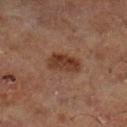The lesion was tiled from a total-body skin photograph and was not biopsied.
A male subject, aged 63–67.
A 15 mm close-up tile from a total-body photography series done for melanoma screening.
Longest diameter approximately 3.5 mm.
The lesion-visualizer software estimated a lesion area of about 7.5 mm², an outline eccentricity of about 0.75 (0 = round, 1 = elongated), and a symmetry-axis asymmetry near 0.2. The software also gave an average lesion color of about L≈28 a*≈17 b*≈24 (CIELAB), roughly 8 lightness units darker than nearby skin, and a lesion-to-skin contrast of about 9 (normalized; higher = more distinct).
Captured under cross-polarized illumination.
The lesion is located on the left lower leg.